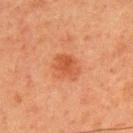Q: Is there a histopathology result?
A: no biopsy performed (imaged during a skin exam)